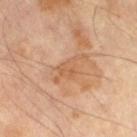Q: What is the anatomic site?
A: the right thigh
Q: Who is the patient?
A: male, about 65 years old
Q: Lesion size?
A: ~5 mm (longest diameter)
Q: What kind of image is this?
A: total-body-photography crop, ~15 mm field of view